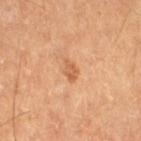Findings:
• follow-up — imaged on a skin check; not biopsied
• anatomic site — the right thigh
• image — ~15 mm crop, total-body skin-cancer survey
• size — ~2.5 mm (longest diameter)
• patient — male, aged around 60